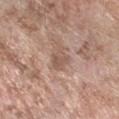workup = imaged on a skin check; not biopsied
acquisition = ~15 mm tile from a whole-body skin photo
patient = female, in their mid-70s
diameter = ~2.5 mm (longest diameter)
lighting = white-light
site = the right forearm